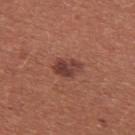{
  "biopsy_status": "not biopsied; imaged during a skin examination",
  "lesion_size": {
    "long_diameter_mm_approx": 3.5
  },
  "site": "chest",
  "image": {
    "source": "total-body photography crop",
    "field_of_view_mm": 15
  },
  "patient": {
    "sex": "female",
    "age_approx": 35
  },
  "lighting": "white-light",
  "automated_metrics": {
    "nevus_likeness_0_100": 90,
    "lesion_detection_confidence_0_100": 100
  }
}